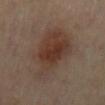The lesion was tiled from a total-body skin photograph and was not biopsied.
On the lower back.
A female patient, roughly 50 years of age.
A roughly 15 mm field-of-view crop from a total-body skin photograph.